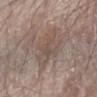Recorded during total-body skin imaging; not selected for excision or biopsy. Cropped from a total-body skin-imaging series; the visible field is about 15 mm. The lesion's longest dimension is about 4.5 mm. The lesion is on the left forearm. A male patient, aged approximately 80.